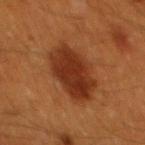biopsy_status: not biopsied; imaged during a skin examination
patient:
  sex: male
  age_approx: 60
lighting: cross-polarized
site: mid back
image:
  source: total-body photography crop
  field_of_view_mm: 15
automated_metrics:
  area_mm2_approx: 20.0
  eccentricity: 0.8
  shape_asymmetry: 0.2
  vs_skin_darker_L: 11.0
  vs_skin_contrast_norm: 10.5
  border_irregularity_0_10: 2.5
  color_variation_0_10: 3.5
  peripheral_color_asymmetry: 1.0
  nevus_likeness_0_100: 100
  lesion_detection_confidence_0_100: 100
lesion_size:
  long_diameter_mm_approx: 6.5